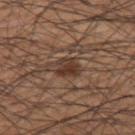image source=total-body-photography crop, ~15 mm field of view
site=the back
illumination=white-light illumination
subject=male, aged around 60